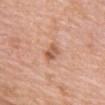Case summary:
• patient: female, approximately 70 years of age
• tile lighting: white-light illumination
• lesion diameter: ~2.5 mm (longest diameter)
• acquisition: total-body-photography crop, ~15 mm field of view
• body site: the front of the torso